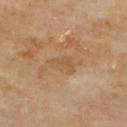{"biopsy_status": "not biopsied; imaged during a skin examination", "site": "upper back", "lighting": "cross-polarized", "lesion_size": {"long_diameter_mm_approx": 3.5}, "patient": {"sex": "male", "age_approx": 65}, "automated_metrics": {"area_mm2_approx": 4.5, "eccentricity": 0.8, "shape_asymmetry": 0.3, "nevus_likeness_0_100": 0, "lesion_detection_confidence_0_100": 100}, "image": {"source": "total-body photography crop", "field_of_view_mm": 15}}